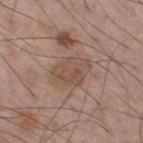  biopsy_status: not biopsied; imaged during a skin examination
  site: right thigh
  lighting: white-light
  patient:
    sex: male
    age_approx: 60
  image:
    source: total-body photography crop
    field_of_view_mm: 15
  automated_metrics:
    area_mm2_approx: 10.0
    shape_asymmetry: 0.2
    border_irregularity_0_10: 2.0
    color_variation_0_10: 3.0